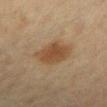| field | value |
|---|---|
| follow-up | imaged on a skin check; not biopsied |
| size | ≈4.5 mm |
| anatomic site | the left lower leg |
| image-analysis metrics | a classifier nevus-likeness of about 90/100 and a detector confidence of about 100 out of 100 that the crop contains a lesion |
| tile lighting | cross-polarized |
| acquisition | ~15 mm tile from a whole-body skin photo |
| subject | female, aged 48 to 52 |